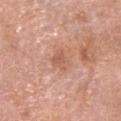Notes:
- notes — no biopsy performed (imaged during a skin exam)
- lesion diameter — about 2.5 mm
- image source — total-body-photography crop, ~15 mm field of view
- body site — the head or neck
- subject — female, approximately 60 years of age